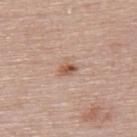notes: no biopsy performed (imaged during a skin exam)
image source: 15 mm crop, total-body photography
lesion diameter: about 3 mm
image-analysis metrics: an area of roughly 5 mm² and a shape eccentricity near 0.65; a lesion color around L≈59 a*≈18 b*≈29 in CIELAB, about 9 CIELAB-L* units darker than the surrounding skin, and a normalized lesion–skin contrast near 6.5; a nevus-likeness score of about 80/100
subject: female, about 50 years old
anatomic site: the upper back